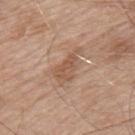| key | value |
|---|---|
| workup | total-body-photography surveillance lesion; no biopsy |
| acquisition | 15 mm crop, total-body photography |
| size | ≈5 mm |
| illumination | white-light |
| patient | male, in their mid-50s |
| anatomic site | the left upper arm |